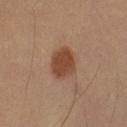biopsy status: catalogued during a skin exam; not biopsied
image-analysis metrics: a footprint of about 9 mm², an outline eccentricity of about 0.65 (0 = round, 1 = elongated), and a shape-asymmetry score of about 0.15 (0 = symmetric); border irregularity of about 1.5 on a 0–10 scale, a within-lesion color-variation index near 2.5/10, and peripheral color asymmetry of about 1
lesion size: ≈4 mm
site: the left forearm
patient: male, in their 40s
lighting: cross-polarized
imaging modality: ~15 mm crop, total-body skin-cancer survey